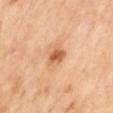Part of a total-body skin-imaging series; this lesion was reviewed on a skin check and was not flagged for biopsy.
From the mid back.
The recorded lesion diameter is about 3 mm.
Captured under cross-polarized illumination.
Cropped from a whole-body photographic skin survey; the tile spans about 15 mm.